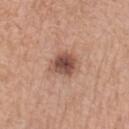Clinical impression: Recorded during total-body skin imaging; not selected for excision or biopsy. Image and clinical context: Cropped from a total-body skin-imaging series; the visible field is about 15 mm. About 3 mm across. Imaged with white-light lighting. The lesion is on the left forearm. A female patient, approximately 55 years of age.